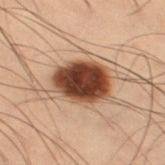Part of a total-body skin-imaging series; this lesion was reviewed on a skin check and was not flagged for biopsy.
A 15 mm close-up tile from a total-body photography series done for melanoma screening.
A male subject aged 53–57.
From the right thigh.